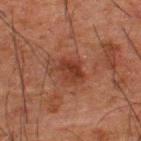| key | value |
|---|---|
| biopsy status | catalogued during a skin exam; not biopsied |
| diameter | ~3 mm (longest diameter) |
| automated metrics | a mean CIELAB color near L≈29 a*≈21 b*≈25, about 7 CIELAB-L* units darker than the surrounding skin, and a normalized lesion–skin contrast near 7.5; border irregularity of about 2 on a 0–10 scale and a within-lesion color-variation index near 3.5/10; a detector confidence of about 100 out of 100 that the crop contains a lesion |
| subject | male, about 50 years old |
| image source | ~15 mm tile from a whole-body skin photo |
| anatomic site | the upper back |